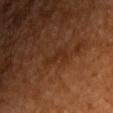biopsy_status: not biopsied; imaged during a skin examination
site: chest
image:
  source: total-body photography crop
  field_of_view_mm: 15
patient:
  sex: male
  age_approx: 60